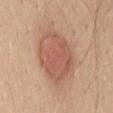patient: female, in their mid- to late 40s | size: ~7 mm (longest diameter) | image: total-body-photography crop, ~15 mm field of view | location: the mid back.